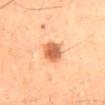No biopsy was performed on this lesion — it was imaged during a full skin examination and was not determined to be concerning. A close-up tile cropped from a whole-body skin photograph, about 15 mm across. A male patient, in their mid-60s. The tile uses cross-polarized illumination. The lesion is located on the back. The lesion-visualizer software estimated a lesion color around L≈52 a*≈23 b*≈34 in CIELAB, a lesion–skin lightness drop of about 14, and a normalized lesion–skin contrast near 9.5. The software also gave an automated nevus-likeness rating near 100 out of 100 and a detector confidence of about 100 out of 100 that the crop contains a lesion.